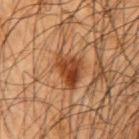workup — catalogued during a skin exam; not biopsied
size — ~4 mm (longest diameter)
body site — the right upper arm
acquisition — ~15 mm tile from a whole-body skin photo
image-analysis metrics — a border-irregularity index near 3.5/10 and a within-lesion color-variation index near 6.5/10
patient — male, aged around 45